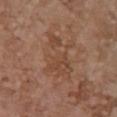workup: imaged on a skin check; not biopsied | lesion size: ≈6 mm | patient: female, about 65 years old | image-analysis metrics: a lesion area of about 17 mm², an eccentricity of roughly 0.8, and a symmetry-axis asymmetry near 0.35; a mean CIELAB color near L≈46 a*≈19 b*≈30, about 6 CIELAB-L* units darker than the surrounding skin, and a normalized lesion–skin contrast near 5; border irregularity of about 6.5 on a 0–10 scale and internal color variation of about 3 on a 0–10 scale; a classifier nevus-likeness of about 0/100 and lesion-presence confidence of about 100/100 | anatomic site: the chest | image source: 15 mm crop, total-body photography.